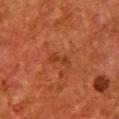Clinical impression: Recorded during total-body skin imaging; not selected for excision or biopsy. Acquisition and patient details: Located on the chest. A roughly 15 mm field-of-view crop from a total-body skin photograph. Imaged with cross-polarized lighting. The recorded lesion diameter is about 3 mm. A female patient, about 50 years old.